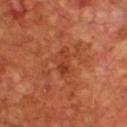• workup · catalogued during a skin exam; not biopsied
• image source · 15 mm crop, total-body photography
• body site · the chest
• subject · male, aged 58–62
• illumination · cross-polarized
• image-analysis metrics · an eccentricity of roughly 0.95 and two-axis asymmetry of about 0.3; a lesion–skin lightness drop of about 7; a border-irregularity index near 3.5/10 and internal color variation of about 1.5 on a 0–10 scale